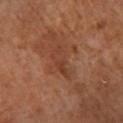Clinical impression: Captured during whole-body skin photography for melanoma surveillance; the lesion was not biopsied. Acquisition and patient details: Captured under cross-polarized illumination. Measured at roughly 4.5 mm in maximum diameter. A female patient in their mid- to late 60s. An algorithmic analysis of the crop reported a footprint of about 6 mm², an eccentricity of roughly 0.9, and a shape-asymmetry score of about 0.35 (0 = symmetric). It also reported a lesion color around L≈39 a*≈23 b*≈30 in CIELAB and roughly 6 lightness units darker than nearby skin. The analysis additionally found border irregularity of about 4.5 on a 0–10 scale, a within-lesion color-variation index near 2.5/10, and peripheral color asymmetry of about 1. It also reported an automated nevus-likeness rating near 0 out of 100 and a detector confidence of about 95 out of 100 that the crop contains a lesion. A 15 mm crop from a total-body photograph taken for skin-cancer surveillance. From the right lower leg.